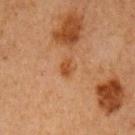| field | value |
|---|---|
| notes | no biopsy performed (imaged during a skin exam) |
| automated metrics | about 7 CIELAB-L* units darker than the surrounding skin and a normalized lesion–skin contrast near 7; lesion-presence confidence of about 100/100 |
| anatomic site | the left upper arm |
| size | ~2 mm (longest diameter) |
| acquisition | total-body-photography crop, ~15 mm field of view |
| subject | female, about 60 years old |